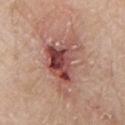{
  "biopsy_status": "not biopsied; imaged during a skin examination",
  "image": {
    "source": "total-body photography crop",
    "field_of_view_mm": 15
  },
  "site": "chest",
  "patient": {
    "sex": "male",
    "age_approx": 80
  },
  "lesion_size": {
    "long_diameter_mm_approx": 7.5
  },
  "lighting": "white-light"
}